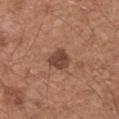The lesion was tiled from a total-body skin photograph and was not biopsied. The lesion is on the arm. Captured under white-light illumination. The recorded lesion diameter is about 3 mm. A male subject approximately 55 years of age. A 15 mm close-up extracted from a 3D total-body photography capture. Automated image analysis of the tile measured a lesion area of about 6 mm², an eccentricity of roughly 0.3, and a shape-asymmetry score of about 0.25 (0 = symmetric). The analysis additionally found border irregularity of about 2.5 on a 0–10 scale, internal color variation of about 2.5 on a 0–10 scale, and a peripheral color-asymmetry measure near 1.